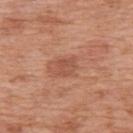Part of a total-body skin-imaging series; this lesion was reviewed on a skin check and was not flagged for biopsy.
A male patient in their 70s.
Captured under white-light illumination.
A 15 mm close-up extracted from a 3D total-body photography capture.
The lesion's longest dimension is about 2.5 mm.
On the upper back.